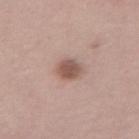Context: The lesion is located on the right thigh. A female subject aged approximately 45. This image is a 15 mm lesion crop taken from a total-body photograph. Automated image analysis of the tile measured a within-lesion color-variation index near 2.5/10 and radial color variation of about 1. And it measured an automated nevus-likeness rating near 90 out of 100 and a lesion-detection confidence of about 100/100. The tile uses white-light illumination.